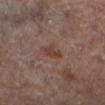Captured during whole-body skin photography for melanoma surveillance; the lesion was not biopsied.
This image is a 15 mm lesion crop taken from a total-body photograph.
The total-body-photography lesion software estimated a footprint of about 4 mm², an eccentricity of roughly 0.7, and a symmetry-axis asymmetry near 0.3. The analysis additionally found a mean CIELAB color near L≈38 a*≈18 b*≈23, roughly 7 lightness units darker than nearby skin, and a normalized border contrast of about 7. And it measured an automated nevus-likeness rating near 0 out of 100 and a detector confidence of about 100 out of 100 that the crop contains a lesion.
The subject is a male about 70 years old.
Located on the left lower leg.
Imaged with cross-polarized lighting.